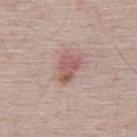Q: Was this lesion biopsied?
A: total-body-photography surveillance lesion; no biopsy
Q: Illumination type?
A: white-light
Q: How large is the lesion?
A: about 3.5 mm
Q: What is the anatomic site?
A: the upper back
Q: Automated lesion metrics?
A: an area of roughly 6 mm² and a shape eccentricity near 0.8; an automated nevus-likeness rating near 15 out of 100 and a detector confidence of about 100 out of 100 that the crop contains a lesion
Q: What is the imaging modality?
A: 15 mm crop, total-body photography
Q: What are the patient's age and sex?
A: male, roughly 50 years of age